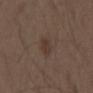Q: Is there a histopathology result?
A: catalogued during a skin exam; not biopsied
Q: How was this image acquired?
A: ~15 mm tile from a whole-body skin photo
Q: Lesion location?
A: the left upper arm
Q: What did automated image analysis measure?
A: about 6 CIELAB-L* units darker than the surrounding skin and a normalized border contrast of about 6; a nevus-likeness score of about 65/100 and a detector confidence of about 100 out of 100 that the crop contains a lesion
Q: Who is the patient?
A: male, aged around 50